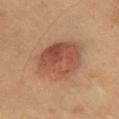Acquisition and patient details:
Approximately 6 mm at its widest. An algorithmic analysis of the crop reported a detector confidence of about 100 out of 100 that the crop contains a lesion. A female subject roughly 55 years of age. The tile uses cross-polarized illumination. A lesion tile, about 15 mm wide, cut from a 3D total-body photograph. The lesion is on the chest.